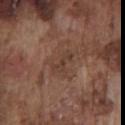* biopsy status: imaged on a skin check; not biopsied
* acquisition: ~15 mm crop, total-body skin-cancer survey
* patient: male, aged around 75
* size: about 3 mm
* lighting: white-light
* anatomic site: the chest